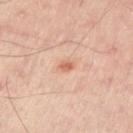  biopsy_status: not biopsied; imaged during a skin examination
  automated_metrics:
    area_mm2_approx: 2.0
    eccentricity: 0.55
    shape_asymmetry: 0.25
    vs_skin_darker_L: 11.0
  site: arm
  patient:
    sex: male
    age_approx: 55
  image:
    source: total-body photography crop
    field_of_view_mm: 15
  lighting: cross-polarized